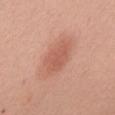Assessment: Recorded during total-body skin imaging; not selected for excision or biopsy. Image and clinical context: The patient is a female roughly 60 years of age. The lesion is located on the back. Automated image analysis of the tile measured a footprint of about 14 mm² and a shape eccentricity near 0.85. A region of skin cropped from a whole-body photographic capture, roughly 15 mm wide.